The lesion is located on the head or neck; approximately 19 mm at its widest; this is a white-light tile; a male subject, aged 58 to 62; a lesion tile, about 15 mm wide, cut from a 3D total-body photograph.
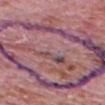Biopsy histopathology demonstrated a nodular basal cell carcinoma (malignant).